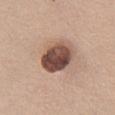Imaged during a routine full-body skin examination; the lesion was not biopsied and no histopathology is available. A female patient, aged 53–57. This is a white-light tile. A close-up tile cropped from a whole-body skin photograph, about 15 mm across. About 5 mm across. From the chest.